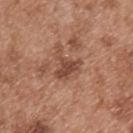notes: total-body-photography surveillance lesion; no biopsy | lighting: white-light illumination | location: the back | lesion size: about 4 mm | acquisition: ~15 mm tile from a whole-body skin photo | automated lesion analysis: an area of roughly 6.5 mm², a shape eccentricity near 0.45, and a shape-asymmetry score of about 0.7 (0 = symmetric); an automated nevus-likeness rating near 5 out of 100 and lesion-presence confidence of about 100/100 | patient: male, in their mid-50s.